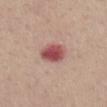The lesion was photographed on a routine skin check and not biopsied; there is no pathology result. On the mid back. The patient is a female in their mid- to late 50s. A 15 mm close-up extracted from a 3D total-body photography capture.